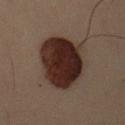- biopsy status — catalogued during a skin exam; not biopsied
- imaging modality — ~15 mm crop, total-body skin-cancer survey
- anatomic site — the left upper arm
- patient — male, in their 40s
- diameter — about 6.5 mm